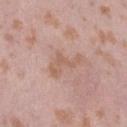Clinical impression:
The lesion was photographed on a routine skin check and not biopsied; there is no pathology result.
Clinical summary:
This image is a 15 mm lesion crop taken from a total-body photograph. Imaged with white-light lighting. The patient is a female roughly 40 years of age. Located on the left thigh.